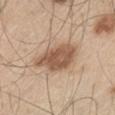Case summary:
– biopsy status · total-body-photography surveillance lesion; no biopsy
– illumination · white-light
– anatomic site · the leg
– diameter · ≈5.5 mm
– patient · male, aged approximately 60
– imaging modality · total-body-photography crop, ~15 mm field of view